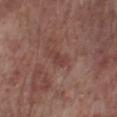Recorded during total-body skin imaging; not selected for excision or biopsy. This is a white-light tile. A lesion tile, about 15 mm wide, cut from a 3D total-body photograph. The lesion-visualizer software estimated a footprint of about 3.5 mm², a shape eccentricity near 0.85, and a symmetry-axis asymmetry near 0.3. The software also gave border irregularity of about 2.5 on a 0–10 scale and internal color variation of about 1 on a 0–10 scale. The recorded lesion diameter is about 2.5 mm. From the right lower leg. A male patient aged around 70.